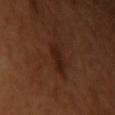No biopsy was performed on this lesion — it was imaged during a full skin examination and was not determined to be concerning.
The total-body-photography lesion software estimated a footprint of about 4.5 mm², an outline eccentricity of about 0.8 (0 = round, 1 = elongated), and two-axis asymmetry of about 0.25. And it measured border irregularity of about 2.5 on a 0–10 scale, a color-variation rating of about 2.5/10, and peripheral color asymmetry of about 1. The analysis additionally found an automated nevus-likeness rating near 15 out of 100 and a detector confidence of about 100 out of 100 that the crop contains a lesion.
A 15 mm close-up tile from a total-body photography series done for melanoma screening.
From the left upper arm.
The subject is a female roughly 55 years of age.